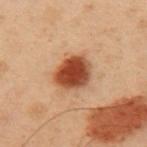biopsy_status: not biopsied; imaged during a skin examination
lesion_size:
  long_diameter_mm_approx: 4.5
automated_metrics:
  cielab_L: 39
  cielab_a: 22
  cielab_b: 30
  vs_skin_darker_L: 15.0
  vs_skin_contrast_norm: 12.0
  nevus_likeness_0_100: 100
site: arm
lighting: cross-polarized
patient:
  sex: male
  age_approx: 55
image:
  source: total-body photography crop
  field_of_view_mm: 15